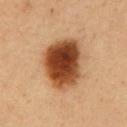Impression:
The lesion was tiled from a total-body skin photograph and was not biopsied.
Image and clinical context:
A male patient, in their 50s. The tile uses cross-polarized illumination. The total-body-photography lesion software estimated an average lesion color of about L≈46 a*≈25 b*≈37 (CIELAB), roughly 21 lightness units darker than nearby skin, and a normalized border contrast of about 14. This image is a 15 mm lesion crop taken from a total-body photograph. The recorded lesion diameter is about 7 mm. The lesion is on the abdomen.